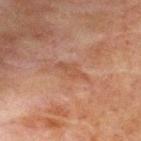The lesion was tiled from a total-body skin photograph and was not biopsied.
From the chest.
A 15 mm close-up tile from a total-body photography series done for melanoma screening.
The lesion-visualizer software estimated a lesion area of about 3.5 mm², an eccentricity of roughly 0.95, and two-axis asymmetry of about 0.35.
A male subject, in their mid- to late 60s.
Captured under cross-polarized illumination.